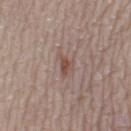Assessment:
Recorded during total-body skin imaging; not selected for excision or biopsy.
Image and clinical context:
The lesion is located on the leg. A 15 mm crop from a total-body photograph taken for skin-cancer surveillance. A female subject aged 58 to 62.